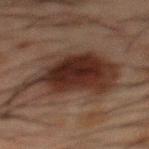Clinical impression:
Recorded during total-body skin imaging; not selected for excision or biopsy.
Acquisition and patient details:
Longest diameter approximately 9 mm. From the mid back. Imaged with cross-polarized lighting. The subject is a male aged approximately 50. A 15 mm crop from a total-body photograph taken for skin-cancer surveillance.